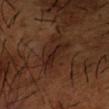Impression: This lesion was catalogued during total-body skin photography and was not selected for biopsy. Image and clinical context: A male subject, aged 63–67. From the right forearm. A 15 mm crop from a total-body photograph taken for skin-cancer surveillance.